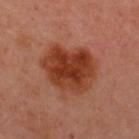biopsy_status: not biopsied; imaged during a skin examination
lighting: cross-polarized
patient:
  sex: male
  age_approx: 50
image:
  source: total-body photography crop
  field_of_view_mm: 15
lesion_size:
  long_diameter_mm_approx: 6.0
site: upper back